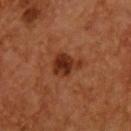Part of a total-body skin-imaging series; this lesion was reviewed on a skin check and was not flagged for biopsy.
The total-body-photography lesion software estimated a footprint of about 7.5 mm² and a shape-asymmetry score of about 0.4 (0 = symmetric). The software also gave a border-irregularity index near 4/10, internal color variation of about 4 on a 0–10 scale, and peripheral color asymmetry of about 1.5.
A male subject aged around 55.
Located on the chest.
A 15 mm close-up tile from a total-body photography series done for melanoma screening.
Approximately 3.5 mm at its widest.
This is a cross-polarized tile.